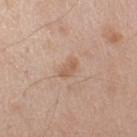No biopsy was performed on this lesion — it was imaged during a full skin examination and was not determined to be concerning.
The subject is a male aged 63–67.
The lesion's longest dimension is about 2.5 mm.
This is a white-light tile.
This image is a 15 mm lesion crop taken from a total-body photograph.
The lesion is located on the right upper arm.